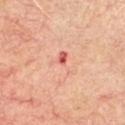Impression: Imaged during a routine full-body skin examination; the lesion was not biopsied and no histopathology is available. Background: A roughly 15 mm field-of-view crop from a total-body skin photograph. This is a cross-polarized tile. A male subject approximately 65 years of age. The lesion is located on the chest. The lesion's longest dimension is about 3.5 mm.